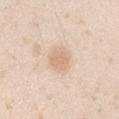No biopsy was performed on this lesion — it was imaged during a full skin examination and was not determined to be concerning.
The patient is a male aged approximately 25.
A region of skin cropped from a whole-body photographic capture, roughly 15 mm wide.
Imaged with white-light lighting.
About 3 mm across.
The lesion is located on the right upper arm.